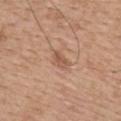workup=catalogued during a skin exam; not biopsied | site=the mid back | subject=male, aged approximately 65 | image source=total-body-photography crop, ~15 mm field of view.